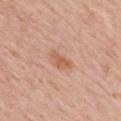Q: Is there a histopathology result?
A: catalogued during a skin exam; not biopsied
Q: How was this image acquired?
A: ~15 mm tile from a whole-body skin photo
Q: How large is the lesion?
A: about 3.5 mm
Q: What lighting was used for the tile?
A: white-light
Q: Lesion location?
A: the upper back
Q: Who is the patient?
A: female, aged approximately 60